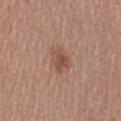{"biopsy_status": "not biopsied; imaged during a skin examination", "lighting": "white-light", "lesion_size": {"long_diameter_mm_approx": 3.0}, "automated_metrics": {"area_mm2_approx": 5.5, "eccentricity": 0.65, "shape_asymmetry": 0.25, "border_irregularity_0_10": 2.5, "color_variation_0_10": 3.0, "peripheral_color_asymmetry": 1.0, "nevus_likeness_0_100": 75, "lesion_detection_confidence_0_100": 100}, "patient": {"sex": "female", "age_approx": 55}, "image": {"source": "total-body photography crop", "field_of_view_mm": 15}, "site": "left thigh"}